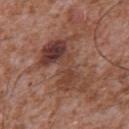Clinical impression: The lesion was tiled from a total-body skin photograph and was not biopsied. Acquisition and patient details: A male patient, approximately 45 years of age. The lesion is located on the front of the torso. Approximately 7 mm at its widest. The tile uses white-light illumination. A region of skin cropped from a whole-body photographic capture, roughly 15 mm wide.